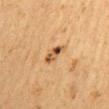The lesion was photographed on a routine skin check and not biopsied; there is no pathology result.
A roughly 15 mm field-of-view crop from a total-body skin photograph.
Captured under cross-polarized illumination.
The subject is a male approximately 60 years of age.
The lesion is on the mid back.
Measured at roughly 3 mm in maximum diameter.